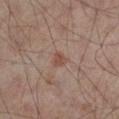{
  "biopsy_status": "not biopsied; imaged during a skin examination",
  "site": "left lower leg",
  "patient": {
    "sex": "male",
    "age_approx": 65
  },
  "image": {
    "source": "total-body photography crop",
    "field_of_view_mm": 15
  },
  "lighting": "cross-polarized",
  "lesion_size": {
    "long_diameter_mm_approx": 2.0
  }
}